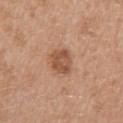Q: Was a biopsy performed?
A: imaged on a skin check; not biopsied
Q: Who is the patient?
A: female, aged 38–42
Q: What is the anatomic site?
A: the right upper arm
Q: How was this image acquired?
A: 15 mm crop, total-body photography
Q: What is the lesion's diameter?
A: ≈3 mm